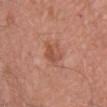Findings:
– follow-up — catalogued during a skin exam; not biopsied
– imaging modality — ~15 mm crop, total-body skin-cancer survey
– site — the chest
– illumination — white-light
– image-analysis metrics — about 8 CIELAB-L* units darker than the surrounding skin and a normalized border contrast of about 6; a border-irregularity rating of about 3/10, a color-variation rating of about 4.5/10, and peripheral color asymmetry of about 1.5
– lesion diameter — about 3 mm
– patient — male, aged around 40